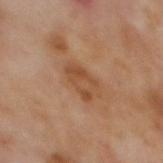Q: Was a biopsy performed?
A: no biopsy performed (imaged during a skin exam)
Q: What is the anatomic site?
A: the mid back
Q: What kind of image is this?
A: ~15 mm crop, total-body skin-cancer survey
Q: How large is the lesion?
A: ~3.5 mm (longest diameter)
Q: How was the tile lit?
A: cross-polarized illumination
Q: Who is the patient?
A: male, approximately 70 years of age